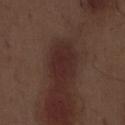The lesion was tiled from a total-body skin photograph and was not biopsied. Captured under white-light illumination. A male patient, aged around 70. Located on the abdomen. The lesion-visualizer software estimated an average lesion color of about L≈25 a*≈19 b*≈19 (CIELAB), a lesion–skin lightness drop of about 6, and a normalized lesion–skin contrast near 7. And it measured border irregularity of about 2.5 on a 0–10 scale and a color-variation rating of about 1/10. This image is a 15 mm lesion crop taken from a total-body photograph. Approximately 3 mm at its widest.